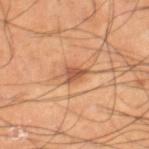• notes · catalogued during a skin exam; not biopsied
• imaging modality · ~15 mm tile from a whole-body skin photo
• patient · male, about 40 years old
• anatomic site · the right lower leg
• lesion size · ~3 mm (longest diameter)
• tile lighting · cross-polarized